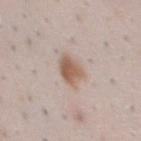Case summary:
– biopsy status · no biopsy performed (imaged during a skin exam)
– location · the front of the torso
– acquisition · ~15 mm tile from a whole-body skin photo
– subject · male, about 50 years old
– illumination · white-light
– automated lesion analysis · a lesion–skin lightness drop of about 11 and a lesion-to-skin contrast of about 8.5 (normalized; higher = more distinct); a classifier nevus-likeness of about 95/100 and a detector confidence of about 100 out of 100 that the crop contains a lesion